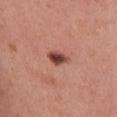follow-up=imaged on a skin check; not biopsied
image=~15 mm crop, total-body skin-cancer survey
TBP lesion metrics=a lesion area of about 4 mm² and a symmetry-axis asymmetry near 0.25; a mean CIELAB color near L≈44 a*≈26 b*≈26, about 17 CIELAB-L* units darker than the surrounding skin, and a normalized border contrast of about 12; a border-irregularity index near 2.5/10, a within-lesion color-variation index near 4/10, and a peripheral color-asymmetry measure near 1; a nevus-likeness score of about 100/100 and a lesion-detection confidence of about 100/100
illumination=white-light illumination
size=about 3 mm
body site=the left thigh
patient=female, roughly 50 years of age